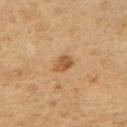<lesion>
  <biopsy_status>not biopsied; imaged during a skin examination</biopsy_status>
  <lesion_size>
    <long_diameter_mm_approx>2.5</long_diameter_mm_approx>
  </lesion_size>
  <image>
    <source>total-body photography crop</source>
    <field_of_view_mm>15</field_of_view_mm>
  </image>
  <patient>
    <sex>female</sex>
    <age_approx>45</age_approx>
  </patient>
  <site>right upper arm</site>
  <automated_metrics>
    <area_mm2_approx>3.5</area_mm2_approx>
    <eccentricity>0.7</eccentricity>
    <shape_asymmetry>0.3</shape_asymmetry>
  </automated_metrics>
</lesion>